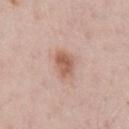Case summary:
– workup: total-body-photography surveillance lesion; no biopsy
– subject: male, in their mid-60s
– site: the chest
– lighting: white-light illumination
– imaging modality: total-body-photography crop, ~15 mm field of view